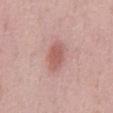<record>
  <biopsy_status>not biopsied; imaged during a skin examination</biopsy_status>
  <automated_metrics>
    <area_mm2_approx>6.5</area_mm2_approx>
    <eccentricity>0.8</eccentricity>
    <shape_asymmetry>0.2</shape_asymmetry>
    <border_irregularity_0_10>2.0</border_irregularity_0_10>
  </automated_metrics>
  <patient>
    <sex>male</sex>
    <age_approx>60</age_approx>
  </patient>
  <site>abdomen</site>
  <image>
    <source>total-body photography crop</source>
    <field_of_view_mm>15</field_of_view_mm>
  </image>
  <lighting>white-light</lighting>
</record>